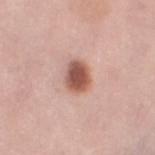{
  "biopsy_status": "not biopsied; imaged during a skin examination",
  "lesion_size": {
    "long_diameter_mm_approx": 3.0
  },
  "automated_metrics": {
    "shape_asymmetry": 0.15
  },
  "lighting": "white-light",
  "site": "left thigh",
  "image": {
    "source": "total-body photography crop",
    "field_of_view_mm": 15
  },
  "patient": {
    "sex": "female",
    "age_approx": 50
  }
}